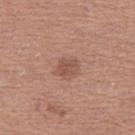notes — imaged on a skin check; not biopsied
location — the right thigh
patient — female, about 50 years old
acquisition — ~15 mm crop, total-body skin-cancer survey